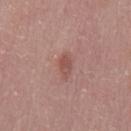Case summary:
– biopsy status — imaged on a skin check; not biopsied
– illumination — white-light illumination
– imaging modality — total-body-photography crop, ~15 mm field of view
– location — the mid back
– lesion size — ~2.5 mm (longest diameter)
– subject — male, approximately 60 years of age